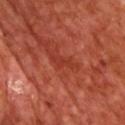biopsy status = no biopsy performed (imaged during a skin exam); location = the chest; size = ≈3 mm; subject = male, approximately 70 years of age; image source = total-body-photography crop, ~15 mm field of view.